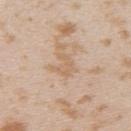Notes:
– biopsy status · imaged on a skin check; not biopsied
– TBP lesion metrics · an average lesion color of about L≈64 a*≈16 b*≈33 (CIELAB), roughly 6 lightness units darker than nearby skin, and a lesion-to-skin contrast of about 5 (normalized; higher = more distinct); a border-irregularity index near 5.5/10, a within-lesion color-variation index near 1/10, and peripheral color asymmetry of about 0.5; a lesion-detection confidence of about 100/100
– location · the upper back
– subject · female, roughly 25 years of age
– imaging modality · total-body-photography crop, ~15 mm field of view
– lesion size · ~3 mm (longest diameter)
– illumination · white-light illumination